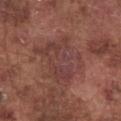biopsy status: catalogued during a skin exam; not biopsied
image source: ~15 mm crop, total-body skin-cancer survey
patient: male, in their mid- to late 70s
location: the chest